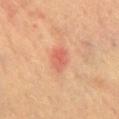A close-up tile cropped from a whole-body skin photograph, about 15 mm across. A female subject, aged approximately 60. Approximately 3.5 mm at its widest. The lesion is on the back. The tile uses cross-polarized illumination.